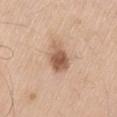Notes:
– biopsy status — total-body-photography surveillance lesion; no biopsy
– tile lighting — white-light
– image — total-body-photography crop, ~15 mm field of view
– location — the back
– image-analysis metrics — an area of roughly 8 mm² and a symmetry-axis asymmetry near 0.15; a border-irregularity rating of about 1.5/10, internal color variation of about 5 on a 0–10 scale, and a peripheral color-asymmetry measure near 2; lesion-presence confidence of about 100/100
– subject — male, about 60 years old
– diameter — ≈3.5 mm